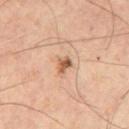subject: male, aged approximately 60 | size: ~2 mm (longest diameter) | anatomic site: the chest | automated lesion analysis: a lesion color around L≈55 a*≈21 b*≈34 in CIELAB, about 14 CIELAB-L* units darker than the surrounding skin, and a lesion-to-skin contrast of about 9.5 (normalized; higher = more distinct); border irregularity of about 3 on a 0–10 scale and radial color variation of about 0.5 | image source: total-body-photography crop, ~15 mm field of view | lighting: cross-polarized illumination.